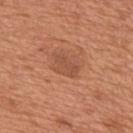| feature | finding |
|---|---|
| follow-up | imaged on a skin check; not biopsied |
| image | ~15 mm crop, total-body skin-cancer survey |
| body site | the upper back |
| patient | male, approximately 65 years of age |
| lesion size | ~3 mm (longest diameter) |